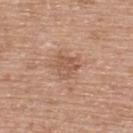The total-body-photography lesion software estimated a footprint of about 6 mm², an eccentricity of roughly 0.55, and two-axis asymmetry of about 0.35. And it measured border irregularity of about 3.5 on a 0–10 scale and a peripheral color-asymmetry measure near 1. The analysis additionally found a lesion-detection confidence of about 100/100. From the upper back. The subject is a female aged 58 to 62. A close-up tile cropped from a whole-body skin photograph, about 15 mm across.